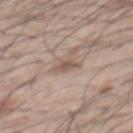{
  "biopsy_status": "not biopsied; imaged during a skin examination",
  "image": {
    "source": "total-body photography crop",
    "field_of_view_mm": 15
  },
  "site": "back",
  "patient": {
    "sex": "male",
    "age_approx": 55
  }
}